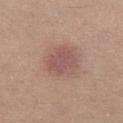Impression:
The lesion was photographed on a routine skin check and not biopsied; there is no pathology result.
Image and clinical context:
The patient is a female in their mid- to late 40s. Located on the right thigh. The tile uses white-light illumination. Automated tile analysis of the lesion measured a lesion area of about 8 mm², an outline eccentricity of about 0.7 (0 = round, 1 = elongated), and a shape-asymmetry score of about 0.2 (0 = symmetric). The analysis additionally found an average lesion color of about L≈52 a*≈22 b*≈22 (CIELAB), roughly 8 lightness units darker than nearby skin, and a normalized lesion–skin contrast near 6.5. Cropped from a total-body skin-imaging series; the visible field is about 15 mm.